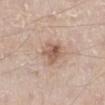This lesion was catalogued during total-body skin photography and was not selected for biopsy. The lesion's longest dimension is about 3 mm. The tile uses white-light illumination. On the left lower leg. The patient is a male about 60 years old. The total-body-photography lesion software estimated an area of roughly 6.5 mm², a shape eccentricity near 0.4, and a symmetry-axis asymmetry near 0.3. The software also gave a mean CIELAB color near L≈57 a*≈18 b*≈28, roughly 11 lightness units darker than nearby skin, and a normalized lesion–skin contrast near 7.5. It also reported a border-irregularity index near 3/10 and a color-variation rating of about 5.5/10. And it measured a classifier nevus-likeness of about 50/100 and a lesion-detection confidence of about 100/100. A 15 mm close-up extracted from a 3D total-body photography capture.